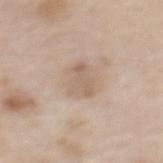Recorded during total-body skin imaging; not selected for excision or biopsy. A 15 mm crop from a total-body photograph taken for skin-cancer surveillance. Longest diameter approximately 4 mm. The lesion is located on the back. The tile uses white-light illumination. The patient is a female about 50 years old.